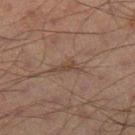| feature | finding |
|---|---|
| biopsy status | total-body-photography surveillance lesion; no biopsy |
| lighting | cross-polarized |
| lesion size | about 3.5 mm |
| acquisition | 15 mm crop, total-body photography |
| location | the right thigh |
| automated lesion analysis | a footprint of about 3.5 mm², an eccentricity of roughly 0.9, and a shape-asymmetry score of about 0.6 (0 = symmetric); a lesion color around L≈35 a*≈14 b*≈21 in CIELAB, about 6 CIELAB-L* units darker than the surrounding skin, and a normalized lesion–skin contrast near 6; a border-irregularity rating of about 7/10, a color-variation rating of about 0/10, and radial color variation of about 0; a classifier nevus-likeness of about 0/100 and a lesion-detection confidence of about 60/100 |
| subject | male, aged 68–72 |